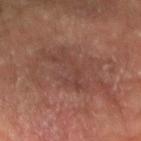| field | value |
|---|---|
| notes | catalogued during a skin exam; not biopsied |
| subject | male, about 65 years old |
| lesion diameter | about 9.5 mm |
| site | the left forearm |
| imaging modality | ~15 mm crop, total-body skin-cancer survey |
| lighting | cross-polarized illumination |